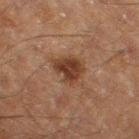workup: imaged on a skin check; not biopsied | body site: the right thigh | patient: male, about 60 years old | tile lighting: cross-polarized | image source: ~15 mm crop, total-body skin-cancer survey | lesion diameter: ~4 mm (longest diameter) | automated metrics: a lesion color around L≈29 a*≈17 b*≈24 in CIELAB, roughly 9 lightness units darker than nearby skin, and a lesion-to-skin contrast of about 8.5 (normalized; higher = more distinct); a nevus-likeness score of about 90/100 and a lesion-detection confidence of about 100/100.